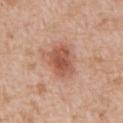Imaged during a routine full-body skin examination; the lesion was not biopsied and no histopathology is available.
Approximately 4 mm at its widest.
Located on the mid back.
The total-body-photography lesion software estimated a border-irregularity rating of about 2/10 and a within-lesion color-variation index near 3.5/10.
A male patient aged 58 to 62.
This image is a 15 mm lesion crop taken from a total-body photograph.
This is a white-light tile.